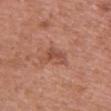{"biopsy_status": "not biopsied; imaged during a skin examination", "site": "chest", "lighting": "white-light", "image": {"source": "total-body photography crop", "field_of_view_mm": 15}, "patient": {"sex": "female", "age_approx": 60}, "lesion_size": {"long_diameter_mm_approx": 3.5}}